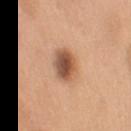Part of a total-body skin-imaging series; this lesion was reviewed on a skin check and was not flagged for biopsy. The tile uses white-light illumination. Automated image analysis of the tile measured a lesion color around L≈55 a*≈21 b*≈33 in CIELAB and roughly 15 lightness units darker than nearby skin. The analysis additionally found a nevus-likeness score of about 95/100 and a lesion-detection confidence of about 100/100. A close-up tile cropped from a whole-body skin photograph, about 15 mm across. From the right upper arm. The recorded lesion diameter is about 4 mm. A female subject, roughly 55 years of age.